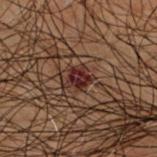The lesion was photographed on a routine skin check and not biopsied; there is no pathology result. The lesion is on the mid back. A male subject aged approximately 50. This image is a 15 mm lesion crop taken from a total-body photograph.